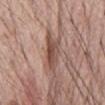No biopsy was performed on this lesion — it was imaged during a full skin examination and was not determined to be concerning.
A male patient in their 70s.
On the abdomen.
A region of skin cropped from a whole-body photographic capture, roughly 15 mm wide.